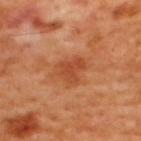Recorded during total-body skin imaging; not selected for excision or biopsy.
Automated image analysis of the tile measured an average lesion color of about L≈48 a*≈30 b*≈39 (CIELAB), roughly 8 lightness units darker than nearby skin, and a normalized lesion–skin contrast near 6.5. It also reported a border-irregularity index near 4/10 and a peripheral color-asymmetry measure near 1. And it measured an automated nevus-likeness rating near 30 out of 100 and a detector confidence of about 100 out of 100 that the crop contains a lesion.
A roughly 15 mm field-of-view crop from a total-body skin photograph.
This is a cross-polarized tile.
The lesion is on the upper back.
Longest diameter approximately 4 mm.
A male patient, aged 48 to 52.